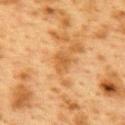Recorded during total-body skin imaging; not selected for excision or biopsy. This is a cross-polarized tile. The recorded lesion diameter is about 2.5 mm. Located on the upper back. A female subject aged around 40. A 15 mm close-up extracted from a 3D total-body photography capture.